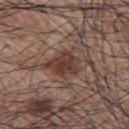The lesion was tiled from a total-body skin photograph and was not biopsied.
Located on the upper back.
A roughly 15 mm field-of-view crop from a total-body skin photograph.
Automated tile analysis of the lesion measured a footprint of about 8 mm², an eccentricity of roughly 0.65, and a symmetry-axis asymmetry near 0.4. And it measured an average lesion color of about L≈38 a*≈19 b*≈22 (CIELAB) and a lesion-to-skin contrast of about 9 (normalized; higher = more distinct). The software also gave an automated nevus-likeness rating near 60 out of 100 and a detector confidence of about 100 out of 100 that the crop contains a lesion.
A male patient, aged 68 to 72.